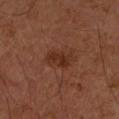biopsy_status: not biopsied; imaged during a skin examination
lesion_size:
  long_diameter_mm_approx: 4.0
lighting: cross-polarized
patient:
  sex: female
  age_approx: 60
image:
  source: total-body photography crop
  field_of_view_mm: 15
site: arm